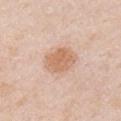| key | value |
|---|---|
| follow-up | catalogued during a skin exam; not biopsied |
| site | the right upper arm |
| subject | male, aged 48 to 52 |
| image | total-body-photography crop, ~15 mm field of view |